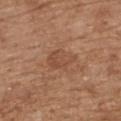The lesion was tiled from a total-body skin photograph and was not biopsied. A region of skin cropped from a whole-body photographic capture, roughly 15 mm wide. The lesion is on the upper back. The recorded lesion diameter is about 4 mm. This is a white-light tile. The patient is a male aged 68 to 72. An algorithmic analysis of the crop reported a footprint of about 7 mm² and a shape-asymmetry score of about 0.35 (0 = symmetric). It also reported a mean CIELAB color near L≈48 a*≈21 b*≈32 and about 7 CIELAB-L* units darker than the surrounding skin. The software also gave border irregularity of about 3.5 on a 0–10 scale and internal color variation of about 2 on a 0–10 scale.